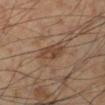  biopsy_status: not biopsied; imaged during a skin examination
  site: left lower leg
  image:
    source: total-body photography crop
    field_of_view_mm: 15
  patient:
    sex: male
    age_approx: 55